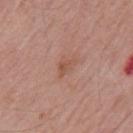subject: male, aged around 65; image source: 15 mm crop, total-body photography; body site: the mid back.